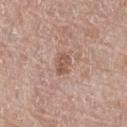The lesion was tiled from a total-body skin photograph and was not biopsied. The lesion's longest dimension is about 3 mm. Automated tile analysis of the lesion measured a border-irregularity rating of about 2.5/10, internal color variation of about 2.5 on a 0–10 scale, and radial color variation of about 1. The software also gave a classifier nevus-likeness of about 0/100 and a detector confidence of about 100 out of 100 that the crop contains a lesion. On the chest. Cropped from a whole-body photographic skin survey; the tile spans about 15 mm. Imaged with white-light lighting. A male subject, aged 68 to 72.